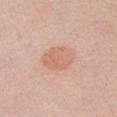| feature | finding |
|---|---|
| site | the chest |
| imaging modality | ~15 mm crop, total-body skin-cancer survey |
| subject | male, approximately 50 years of age |
| image-analysis metrics | a mean CIELAB color near L≈65 a*≈22 b*≈30, about 7 CIELAB-L* units darker than the surrounding skin, and a normalized lesion–skin contrast near 5.5; border irregularity of about 1.5 on a 0–10 scale, a color-variation rating of about 4/10, and peripheral color asymmetry of about 1.5 |
| illumination | white-light illumination |
| lesion diameter | ≈4.5 mm |